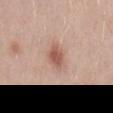<tbp_lesion>
  <biopsy_status>not biopsied; imaged during a skin examination</biopsy_status>
  <lesion_size>
    <long_diameter_mm_approx>3.0</long_diameter_mm_approx>
  </lesion_size>
  <image>
    <source>total-body photography crop</source>
    <field_of_view_mm>15</field_of_view_mm>
  </image>
  <patient>
    <sex>male</sex>
    <age_approx>40</age_approx>
  </patient>
  <lighting>white-light</lighting>
  <automated_metrics>
    <area_mm2_approx>5.5</area_mm2_approx>
    <eccentricity>0.7</eccentricity>
    <shape_asymmetry>0.25</shape_asymmetry>
    <cielab_L>56</cielab_L>
    <cielab_a>22</cielab_a>
    <cielab_b>27</cielab_b>
    <vs_skin_darker_L>11.0</vs_skin_darker_L>
    <vs_skin_contrast_norm>7.5</vs_skin_contrast_norm>
    <nevus_likeness_0_100>85</nevus_likeness_0_100>
    <lesion_detection_confidence_0_100>100</lesion_detection_confidence_0_100>
  </automated_metrics>
  <site>mid back</site>
</tbp_lesion>